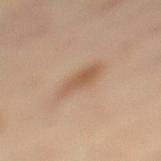Case summary:
- follow-up · catalogued during a skin exam; not biopsied
- image · 15 mm crop, total-body photography
- size · about 4.5 mm
- TBP lesion metrics · an outline eccentricity of about 0.7 (0 = round, 1 = elongated) and a shape-asymmetry score of about 0.2 (0 = symmetric); border irregularity of about 2 on a 0–10 scale and peripheral color asymmetry of about 1
- patient · female, aged 53 to 57
- location · the left lower leg